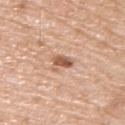A male subject roughly 65 years of age. Cropped from a total-body skin-imaging series; the visible field is about 15 mm. Imaged with white-light lighting. The lesion is located on the upper back.